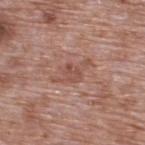{"biopsy_status": "not biopsied; imaged during a skin examination", "patient": {"sex": "male", "age_approx": 70}, "site": "upper back", "lesion_size": {"long_diameter_mm_approx": 5.0}, "image": {"source": "total-body photography crop", "field_of_view_mm": 15}}